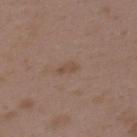Part of a total-body skin-imaging series; this lesion was reviewed on a skin check and was not flagged for biopsy.
A lesion tile, about 15 mm wide, cut from a 3D total-body photograph.
A female subject, approximately 35 years of age.
On the upper back.